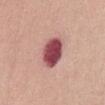– workup: catalogued during a skin exam; not biopsied
– lesion diameter: ~4 mm (longest diameter)
– anatomic site: the abdomen
– patient: female, roughly 55 years of age
– imaging modality: total-body-photography crop, ~15 mm field of view
– automated lesion analysis: an average lesion color of about L≈49 a*≈31 b*≈20 (CIELAB), a lesion–skin lightness drop of about 20, and a normalized border contrast of about 13.5; internal color variation of about 5.5 on a 0–10 scale and peripheral color asymmetry of about 1.5